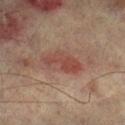Impression: No biopsy was performed on this lesion — it was imaged during a full skin examination and was not determined to be concerning. Image and clinical context: A male patient aged 73 to 77. On the right lower leg. Cropped from a whole-body photographic skin survey; the tile spans about 15 mm.